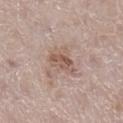follow-up: no biopsy performed (imaged during a skin exam)
lighting: white-light
size: ≈3.5 mm
image source: total-body-photography crop, ~15 mm field of view
TBP lesion metrics: a lesion area of about 7 mm², an outline eccentricity of about 0.7 (0 = round, 1 = elongated), and a shape-asymmetry score of about 0.3 (0 = symmetric)
body site: the leg
patient: female, aged around 50